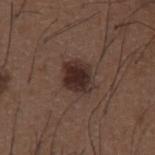Notes:
* workup: imaged on a skin check; not biopsied
* imaging modality: total-body-photography crop, ~15 mm field of view
* patient: male, aged approximately 50
* tile lighting: white-light illumination
* location: the abdomen